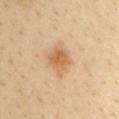notes=no biopsy performed (imaged during a skin exam); illumination=cross-polarized; patient=male, roughly 40 years of age; body site=the left upper arm; acquisition=15 mm crop, total-body photography.